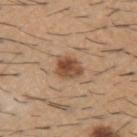notes=imaged on a skin check; not biopsied
illumination=white-light
patient=male, roughly 60 years of age
lesion size=≈3.5 mm
anatomic site=the upper back
automated metrics=an area of roughly 7.5 mm², a shape eccentricity near 0.7, and two-axis asymmetry of about 0.2; about 13 CIELAB-L* units darker than the surrounding skin and a normalized lesion–skin contrast near 9; a border-irregularity rating of about 2/10, a color-variation rating of about 5/10, and radial color variation of about 1.5; a detector confidence of about 100 out of 100 that the crop contains a lesion
image source=~15 mm crop, total-body skin-cancer survey